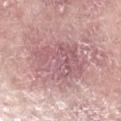notes: total-body-photography surveillance lesion; no biopsy | subject: female, aged 68 to 72 | imaging modality: total-body-photography crop, ~15 mm field of view | anatomic site: the left forearm | lighting: white-light | diameter: ≈6.5 mm | automated lesion analysis: a mean CIELAB color near L≈58 a*≈24 b*≈17, a lesion–skin lightness drop of about 9, and a lesion-to-skin contrast of about 6 (normalized; higher = more distinct); an automated nevus-likeness rating near 0 out of 100.